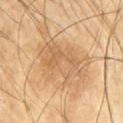This image is a 15 mm lesion crop taken from a total-body photograph.
A male patient aged 58–62.
The lesion is on the right upper arm.